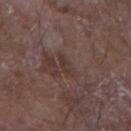Case summary:
• notes — catalogued during a skin exam; not biopsied
• image — total-body-photography crop, ~15 mm field of view
• patient — male, in their mid- to late 60s
• location — the left forearm
• lesion diameter — ~3 mm (longest diameter)